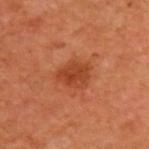Impression: Part of a total-body skin-imaging series; this lesion was reviewed on a skin check and was not flagged for biopsy. Image and clinical context: The lesion is located on the back. The patient is a male about 60 years old. This is a cross-polarized tile. A close-up tile cropped from a whole-body skin photograph, about 15 mm across. Measured at roughly 4 mm in maximum diameter.